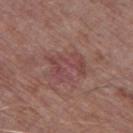Captured during whole-body skin photography for melanoma surveillance; the lesion was not biopsied. The subject is a male aged around 75. About 4 mm across. The lesion is on the left thigh. An algorithmic analysis of the crop reported an area of roughly 10 mm². It also reported a border-irregularity rating of about 7.5/10, a color-variation rating of about 3/10, and peripheral color asymmetry of about 1. And it measured a nevus-likeness score of about 0/100 and a lesion-detection confidence of about 95/100. Cropped from a total-body skin-imaging series; the visible field is about 15 mm.